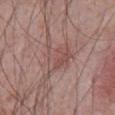biopsy status: catalogued during a skin exam; not biopsied
subject: male, approximately 70 years of age
acquisition: ~15 mm crop, total-body skin-cancer survey
anatomic site: the abdomen